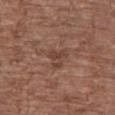Q: Is there a histopathology result?
A: imaged on a skin check; not biopsied
Q: Lesion size?
A: about 3 mm
Q: Where on the body is the lesion?
A: the left thigh
Q: Who is the patient?
A: female, in their mid- to late 70s
Q: What kind of image is this?
A: ~15 mm crop, total-body skin-cancer survey